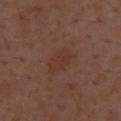Part of a total-body skin-imaging series; this lesion was reviewed on a skin check and was not flagged for biopsy. A male subject aged 48–52. A roughly 15 mm field-of-view crop from a total-body skin photograph. Approximately 4 mm at its widest. Imaged with white-light lighting.